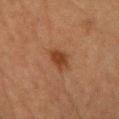The lesion was photographed on a routine skin check and not biopsied; there is no pathology result. A female patient in their mid- to late 50s. A region of skin cropped from a whole-body photographic capture, roughly 15 mm wide. Automated image analysis of the tile measured an area of roughly 4.5 mm² and a shape-asymmetry score of about 0.3 (0 = symmetric). It also reported a mean CIELAB color near L≈35 a*≈21 b*≈30, a lesion–skin lightness drop of about 9, and a normalized lesion–skin contrast near 8.5. The analysis additionally found a detector confidence of about 100 out of 100 that the crop contains a lesion. This is a cross-polarized tile. The lesion's longest dimension is about 3 mm. The lesion is on the front of the torso.